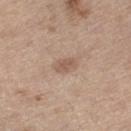Imaged during a routine full-body skin examination; the lesion was not biopsied and no histopathology is available. A region of skin cropped from a whole-body photographic capture, roughly 15 mm wide. The lesion is located on the left thigh. A male subject roughly 70 years of age. Measured at roughly 2.5 mm in maximum diameter. Automated tile analysis of the lesion measured a lesion area of about 3.5 mm², an eccentricity of roughly 0.8, and two-axis asymmetry of about 0.25. It also reported a border-irregularity rating of about 2/10 and a within-lesion color-variation index near 1/10. Imaged with white-light lighting.